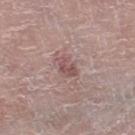Context: A male patient, approximately 80 years of age. This is a white-light tile. The lesion is located on the right thigh. A 15 mm close-up extracted from a 3D total-body photography capture. Longest diameter approximately 2.5 mm.